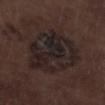Clinical impression:
Imaged during a routine full-body skin examination; the lesion was not biopsied and no histopathology is available.
Image and clinical context:
A male patient, approximately 70 years of age. Measured at roughly 8 mm in maximum diameter. On the left lower leg. A region of skin cropped from a whole-body photographic capture, roughly 15 mm wide. Imaged with white-light lighting.